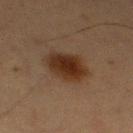workup: total-body-photography surveillance lesion; no biopsy
image source: total-body-photography crop, ~15 mm field of view
subject: female, aged 53–57
anatomic site: the left thigh